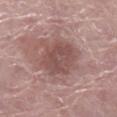Notes:
– notes · imaged on a skin check; not biopsied
– subject · female, roughly 60 years of age
– location · the left lower leg
– imaging modality · 15 mm crop, total-body photography